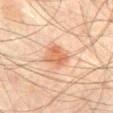anatomic site — the abdomen | image source — ~15 mm tile from a whole-body skin photo | subject — male, aged around 65 | size — ≈3.5 mm | lighting — cross-polarized illumination | automated metrics — an outline eccentricity of about 0.6 (0 = round, 1 = elongated) and two-axis asymmetry of about 0.25; about 11 CIELAB-L* units darker than the surrounding skin and a lesion-to-skin contrast of about 7 (normalized; higher = more distinct); a border-irregularity rating of about 2.5/10, a color-variation rating of about 3.5/10, and radial color variation of about 1.